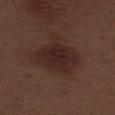Q: Is there a histopathology result?
A: total-body-photography surveillance lesion; no biopsy
Q: Lesion location?
A: the leg
Q: Illumination type?
A: white-light
Q: What kind of image is this?
A: ~15 mm tile from a whole-body skin photo
Q: Patient demographics?
A: male, approximately 70 years of age